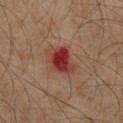No biopsy was performed on this lesion — it was imaged during a full skin examination and was not determined to be concerning.
A male patient roughly 60 years of age.
The tile uses cross-polarized illumination.
The lesion is on the abdomen.
A close-up tile cropped from a whole-body skin photograph, about 15 mm across.
About 4 mm across.